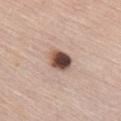Recorded during total-body skin imaging; not selected for excision or biopsy. The patient is a female aged 63 to 67. On the chest. Imaged with white-light lighting. Measured at roughly 3 mm in maximum diameter. A region of skin cropped from a whole-body photographic capture, roughly 15 mm wide.